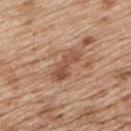diameter = ~4.5 mm (longest diameter) | location = the upper back | acquisition = total-body-photography crop, ~15 mm field of view | subject = male, roughly 70 years of age | illumination = white-light illumination | TBP lesion metrics = an area of roughly 7 mm² and a symmetry-axis asymmetry near 0.35.